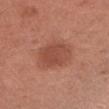subject — female, in their 50s; illumination — white-light illumination; diameter — ~4 mm (longest diameter); acquisition — ~15 mm tile from a whole-body skin photo; site — the left forearm.